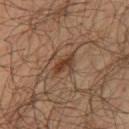Findings:
• notes: catalogued during a skin exam; not biopsied
• image source: ~15 mm tile from a whole-body skin photo
• anatomic site: the right thigh
• illumination: cross-polarized
• patient: male, aged 63 to 67